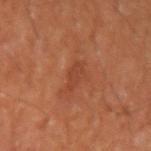Imaged during a routine full-body skin examination; the lesion was not biopsied and no histopathology is available.